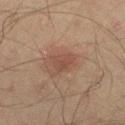Impression: The lesion was tiled from a total-body skin photograph and was not biopsied. Clinical summary: Measured at roughly 3 mm in maximum diameter. This is a cross-polarized tile. This image is a 15 mm lesion crop taken from a total-body photograph. The patient is a male aged approximately 45. On the right thigh.